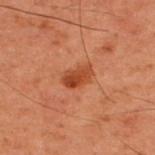follow-up: total-body-photography surveillance lesion; no biopsy | lighting: cross-polarized illumination | image: 15 mm crop, total-body photography | patient: male, aged 58 to 62 | site: the upper back | image-analysis metrics: a lesion area of about 5.5 mm², an outline eccentricity of about 0.8 (0 = round, 1 = elongated), and a shape-asymmetry score of about 0.2 (0 = symmetric).